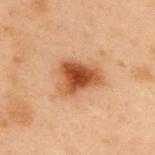The lesion was tiled from a total-body skin photograph and was not biopsied.
This is a cross-polarized tile.
A 15 mm close-up extracted from a 3D total-body photography capture.
The subject is a male about 55 years old.
The lesion-visualizer software estimated a nevus-likeness score of about 100/100 and a lesion-detection confidence of about 100/100.
Approximately 5 mm at its widest.
On the upper back.